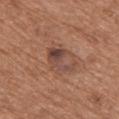| feature | finding |
|---|---|
| follow-up | imaged on a skin check; not biopsied |
| anatomic site | the upper back |
| imaging modality | ~15 mm crop, total-body skin-cancer survey |
| patient | male, aged 68–72 |